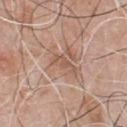The lesion was tiled from a total-body skin photograph and was not biopsied.
A lesion tile, about 15 mm wide, cut from a 3D total-body photograph.
The patient is a male aged 68–72.
The tile uses white-light illumination.
The lesion-visualizer software estimated about 8 CIELAB-L* units darker than the surrounding skin. The software also gave a border-irregularity index near 5.5/10, a color-variation rating of about 1/10, and radial color variation of about 0. It also reported an automated nevus-likeness rating near 0 out of 100 and a detector confidence of about 90 out of 100 that the crop contains a lesion.
The recorded lesion diameter is about 3.5 mm.
The lesion is on the front of the torso.